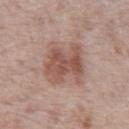biopsy status = imaged on a skin check; not biopsied | automated metrics = a lesion area of about 17 mm², an eccentricity of roughly 0.4, and a symmetry-axis asymmetry near 0.3; a lesion–skin lightness drop of about 10 and a normalized border contrast of about 7.5; an automated nevus-likeness rating near 55 out of 100 and a detector confidence of about 100 out of 100 that the crop contains a lesion | image = ~15 mm tile from a whole-body skin photo | location = the abdomen | patient = male, approximately 70 years of age | tile lighting = white-light.